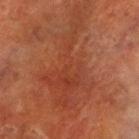<tbp_lesion>
<biopsy_status>not biopsied; imaged during a skin examination</biopsy_status>
<image>
  <source>total-body photography crop</source>
  <field_of_view_mm>15</field_of_view_mm>
</image>
<lighting>cross-polarized</lighting>
<patient>
  <sex>male</sex>
  <age_approx>70</age_approx>
</patient>
<site>right lower leg</site>
<automated_metrics>
  <cielab_L>34</cielab_L>
  <cielab_a>25</cielab_a>
  <cielab_b>29</cielab_b>
  <vs_skin_darker_L>6.0</vs_skin_darker_L>
  <border_irregularity_0_10>9.5</border_irregularity_0_10>
  <color_variation_0_10>3.0</color_variation_0_10>
  <peripheral_color_asymmetry>1.0</peripheral_color_asymmetry>
  <nevus_likeness_0_100>0</nevus_likeness_0_100>
  <lesion_detection_confidence_0_100>95</lesion_detection_confidence_0_100>
</automated_metrics>
<lesion_size>
  <long_diameter_mm_approx>11.0</long_diameter_mm_approx>
</lesion_size>
</tbp_lesion>